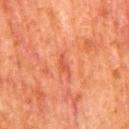workup = imaged on a skin check; not biopsied | lesion size = about 2.5 mm | illumination = cross-polarized illumination | subject = male, aged 78 to 82 | body site = the mid back | TBP lesion metrics = an area of roughly 2 mm², a shape eccentricity near 0.9, and a symmetry-axis asymmetry near 0.5; a lesion-detection confidence of about 100/100 | imaging modality = total-body-photography crop, ~15 mm field of view.